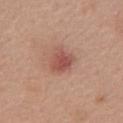A female patient aged around 60. The total-body-photography lesion software estimated a footprint of about 7 mm², an eccentricity of roughly 0.65, and two-axis asymmetry of about 0.25. The software also gave an average lesion color of about L≈52 a*≈26 b*≈26 (CIELAB) and a lesion–skin lightness drop of about 10. The software also gave a border-irregularity rating of about 2.5/10, a color-variation rating of about 3/10, and a peripheral color-asymmetry measure near 1. A region of skin cropped from a whole-body photographic capture, roughly 15 mm wide. Located on the chest. Measured at roughly 3.5 mm in maximum diameter.